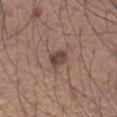| feature | finding |
|---|---|
| workup | no biopsy performed (imaged during a skin exam) |
| location | the right forearm |
| subject | male, about 60 years old |
| illumination | white-light illumination |
| lesion size | about 2.5 mm |
| image | total-body-photography crop, ~15 mm field of view |